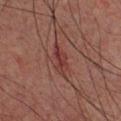Q: Is there a histopathology result?
A: total-body-photography surveillance lesion; no biopsy